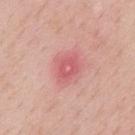Case summary:
– notes · no biopsy performed (imaged during a skin exam)
– location · the mid back
– size · ~3.5 mm (longest diameter)
– automated metrics · an area of roughly 7.5 mm², a shape eccentricity near 0.65, and two-axis asymmetry of about 0.2; a mean CIELAB color near L≈60 a*≈33 b*≈24, roughly 9 lightness units darker than nearby skin, and a lesion-to-skin contrast of about 6 (normalized; higher = more distinct); a classifier nevus-likeness of about 0/100 and lesion-presence confidence of about 100/100
– tile lighting · white-light illumination
– patient · male, in their mid- to late 50s
– image source · total-body-photography crop, ~15 mm field of view